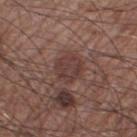Q: What is the anatomic site?
A: the right thigh
Q: What are the patient's age and sex?
A: male, aged 58 to 62
Q: What lighting was used for the tile?
A: white-light illumination
Q: How was this image acquired?
A: ~15 mm crop, total-body skin-cancer survey
Q: Automated lesion metrics?
A: a lesion area of about 8.5 mm², a shape eccentricity near 0.35, and a shape-asymmetry score of about 0.15 (0 = symmetric); a classifier nevus-likeness of about 0/100 and a lesion-detection confidence of about 95/100
Q: Lesion size?
A: ~3.5 mm (longest diameter)